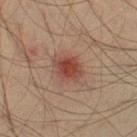{
  "image": {
    "source": "total-body photography crop",
    "field_of_view_mm": 15
  },
  "site": "right thigh",
  "lesion_size": {
    "long_diameter_mm_approx": 3.5
  },
  "lighting": "cross-polarized",
  "patient": {
    "sex": "male",
    "age_approx": 45
  }
}